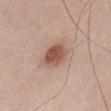notes: catalogued during a skin exam; not biopsied | image source: total-body-photography crop, ~15 mm field of view | patient: male, aged 48 to 52 | anatomic site: the front of the torso | lesion size: ~3.5 mm (longest diameter).